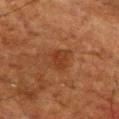– follow-up: total-body-photography surveillance lesion; no biopsy
– body site: the chest
– image source: ~15 mm tile from a whole-body skin photo
– subject: male, roughly 65 years of age